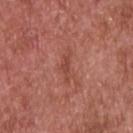Findings:
• follow-up: no biopsy performed (imaged during a skin exam)
• location: the chest
• lesion diameter: ≈4 mm
• patient: male, approximately 65 years of age
• automated lesion analysis: a lesion area of about 5 mm² and a symmetry-axis asymmetry near 0.4; a color-variation rating of about 2/10 and a peripheral color-asymmetry measure near 0.5
• illumination: white-light
• image source: 15 mm crop, total-body photography